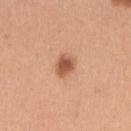Case summary:
- subject · female, aged approximately 30
- image · ~15 mm tile from a whole-body skin photo
- site · the right upper arm